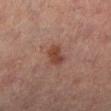The lesion was tiled from a total-body skin photograph and was not biopsied.
The recorded lesion diameter is about 3 mm.
Automated image analysis of the tile measured an area of roughly 5 mm², an outline eccentricity of about 0.85 (0 = round, 1 = elongated), and a shape-asymmetry score of about 0.25 (0 = symmetric). The software also gave a mean CIELAB color near L≈35 a*≈20 b*≈23, about 8 CIELAB-L* units darker than the surrounding skin, and a normalized lesion–skin contrast near 8. The analysis additionally found a border-irregularity rating of about 2.5/10 and internal color variation of about 3 on a 0–10 scale.
The lesion is located on the left lower leg.
A female subject, roughly 70 years of age.
Captured under cross-polarized illumination.
A lesion tile, about 15 mm wide, cut from a 3D total-body photograph.